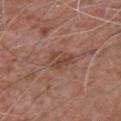Impression: Recorded during total-body skin imaging; not selected for excision or biopsy. Context: The recorded lesion diameter is about 3.5 mm. A male patient, in their 60s. Located on the back. Captured under white-light illumination. A close-up tile cropped from a whole-body skin photograph, about 15 mm across.